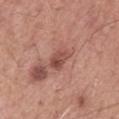Impression:
Recorded during total-body skin imaging; not selected for excision or biopsy.
Acquisition and patient details:
A male patient, aged 53–57. On the mid back. Cropped from a whole-body photographic skin survey; the tile spans about 15 mm. The tile uses white-light illumination. The recorded lesion diameter is about 3 mm.